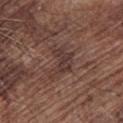| key | value |
|---|---|
| notes | imaged on a skin check; not biopsied |
| imaging modality | total-body-photography crop, ~15 mm field of view |
| subject | male, in their 70s |
| site | the left forearm |